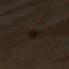notes: no biopsy performed (imaged during a skin exam)
location: the front of the torso
lesion size: ≈4 mm
lighting: cross-polarized
subject: female, aged 53 to 57
image: 15 mm crop, total-body photography